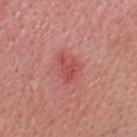Notes:
• workup · no biopsy performed (imaged during a skin exam)
• body site · the head or neck
• automated metrics · a lesion area of about 5 mm², a shape eccentricity near 0.8, and a symmetry-axis asymmetry near 0.5; a lesion color around L≈51 a*≈34 b*≈27 in CIELAB, roughly 9 lightness units darker than nearby skin, and a normalized border contrast of about 6.5; a border-irregularity rating of about 5.5/10 and a within-lesion color-variation index near 1.5/10
• image source · 15 mm crop, total-body photography
• patient · male, aged 28–32
• lighting · white-light illumination